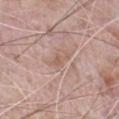<tbp_lesion>
  <biopsy_status>not biopsied; imaged during a skin examination</biopsy_status>
  <site>chest</site>
  <lesion_size>
    <long_diameter_mm_approx>2.5</long_diameter_mm_approx>
  </lesion_size>
  <image>
    <source>total-body photography crop</source>
    <field_of_view_mm>15</field_of_view_mm>
  </image>
  <lighting>white-light</lighting>
  <automated_metrics>
    <cielab_L>58</cielab_L>
    <cielab_a>18</cielab_a>
    <cielab_b>26</cielab_b>
    <vs_skin_darker_L>7.0</vs_skin_darker_L>
    <vs_skin_contrast_norm>5.0</vs_skin_contrast_norm>
    <border_irregularity_0_10>4.0</border_irregularity_0_10>
    <color_variation_0_10>0.5</color_variation_0_10>
    <peripheral_color_asymmetry>0.0</peripheral_color_asymmetry>
  </automated_metrics>
  <patient>
    <sex>male</sex>
    <age_approx>70</age_approx>
  </patient>
</tbp_lesion>